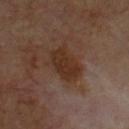Captured during whole-body skin photography for melanoma surveillance; the lesion was not biopsied.
The tile uses cross-polarized illumination.
On the chest.
A region of skin cropped from a whole-body photographic capture, roughly 15 mm wide.
The lesion-visualizer software estimated a footprint of about 9 mm² and a symmetry-axis asymmetry near 0.2. And it measured an average lesion color of about L≈28 a*≈17 b*≈25 (CIELAB), about 7 CIELAB-L* units darker than the surrounding skin, and a lesion-to-skin contrast of about 8.5 (normalized; higher = more distinct). The analysis additionally found a border-irregularity rating of about 2.5/10, a color-variation rating of about 3/10, and peripheral color asymmetry of about 1.
The subject is a male aged 63–67.